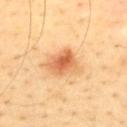Q: Is there a histopathology result?
A: no biopsy performed (imaged during a skin exam)